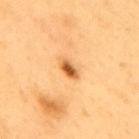Clinical impression: Captured during whole-body skin photography for melanoma surveillance; the lesion was not biopsied. Context: A male subject, in their 60s. Imaged with cross-polarized lighting. The lesion's longest dimension is about 2.5 mm. Located on the back. This image is a 15 mm lesion crop taken from a total-body photograph.